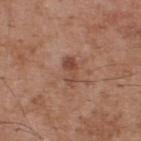Imaged during a routine full-body skin examination; the lesion was not biopsied and no histopathology is available.
This is a white-light tile.
A male patient, aged 53–57.
From the back.
A 15 mm crop from a total-body photograph taken for skin-cancer surveillance.
Automated tile analysis of the lesion measured an area of roughly 4.5 mm² and an outline eccentricity of about 0.85 (0 = round, 1 = elongated). The analysis additionally found a lesion color around L≈47 a*≈22 b*≈28 in CIELAB, roughly 9 lightness units darker than nearby skin, and a lesion-to-skin contrast of about 7 (normalized; higher = more distinct). The software also gave a border-irregularity index near 4/10 and internal color variation of about 3 on a 0–10 scale. And it measured a nevus-likeness score of about 0/100 and lesion-presence confidence of about 100/100.